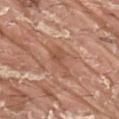Clinical impression: Recorded during total-body skin imaging; not selected for excision or biopsy. Clinical summary: The recorded lesion diameter is about 4 mm. The lesion is located on the right upper arm. The tile uses white-light illumination. Automated image analysis of the tile measured a lesion area of about 5.5 mm² and an outline eccentricity of about 0.9 (0 = round, 1 = elongated). The software also gave an average lesion color of about L≈53 a*≈23 b*≈32 (CIELAB), roughly 8 lightness units darker than nearby skin, and a normalized lesion–skin contrast near 5.5. A 15 mm close-up extracted from a 3D total-body photography capture. The patient is a male aged 38 to 42.